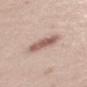notes = imaged on a skin check; not biopsied
subject = male, in their mid-20s
lighting = white-light illumination
lesion size = ~4.5 mm (longest diameter)
body site = the mid back
image source = ~15 mm crop, total-body skin-cancer survey